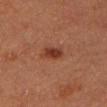Findings:
* biopsy status: total-body-photography surveillance lesion; no biopsy
* illumination: cross-polarized illumination
* acquisition: 15 mm crop, total-body photography
* site: the head or neck
* lesion diameter: ~2.5 mm (longest diameter)
* patient: female, aged 68 to 72